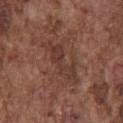Automated image analysis of the tile measured an average lesion color of about L≈37 a*≈21 b*≈24 (CIELAB) and a normalized border contrast of about 6. The software also gave a border-irregularity rating of about 7/10, a color-variation rating of about 2.5/10, and radial color variation of about 1. The lesion is located on the front of the torso. A roughly 15 mm field-of-view crop from a total-body skin photograph. The tile uses white-light illumination. The subject is a male aged 73 to 77. The recorded lesion diameter is about 6 mm.